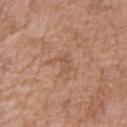This lesion was catalogued during total-body skin photography and was not selected for biopsy.
The recorded lesion diameter is about 3 mm.
A 15 mm close-up tile from a total-body photography series done for melanoma screening.
A female subject, aged 53 to 57.
The lesion is on the right upper arm.
Automated image analysis of the tile measured a lesion area of about 3.5 mm², an eccentricity of roughly 0.8, and two-axis asymmetry of about 0.45. The software also gave a border-irregularity rating of about 6/10, a within-lesion color-variation index near 0/10, and radial color variation of about 0. It also reported an automated nevus-likeness rating near 0 out of 100.